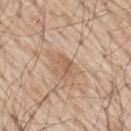Q: Was this lesion biopsied?
A: catalogued during a skin exam; not biopsied
Q: What is the lesion's diameter?
A: ~2.5 mm (longest diameter)
Q: Illumination type?
A: white-light
Q: Who is the patient?
A: male, aged 68–72
Q: What is the imaging modality?
A: 15 mm crop, total-body photography
Q: What is the anatomic site?
A: the mid back
Q: What did automated image analysis measure?
A: border irregularity of about 3.5 on a 0–10 scale and a peripheral color-asymmetry measure near 0.5